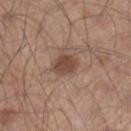workup: imaged on a skin check; not biopsied
size: ≈3.5 mm
acquisition: ~15 mm tile from a whole-body skin photo
body site: the right lower leg
subject: male, aged 28–32
lighting: white-light illumination